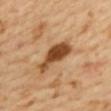Findings:
* workup: catalogued during a skin exam; not biopsied
* patient: female, in their mid- to late 50s
* tile lighting: cross-polarized illumination
* acquisition: 15 mm crop, total-body photography
* anatomic site: the mid back
* automated lesion analysis: a lesion area of about 10 mm²; a lesion color around L≈49 a*≈23 b*≈39 in CIELAB, a lesion–skin lightness drop of about 17, and a normalized border contrast of about 11.5; a nevus-likeness score of about 25/100 and a detector confidence of about 100 out of 100 that the crop contains a lesion